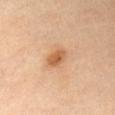Acquisition and patient details: A 15 mm close-up tile from a total-body photography series done for melanoma screening. The tile uses cross-polarized illumination. The lesion's longest dimension is about 3 mm. A male patient aged 33 to 37. On the chest.